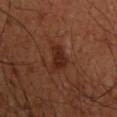| field | value |
|---|---|
| biopsy status | total-body-photography surveillance lesion; no biopsy |
| anatomic site | the left upper arm |
| image | total-body-photography crop, ~15 mm field of view |
| lesion diameter | ≈3.5 mm |
| illumination | cross-polarized |
| subject | male, aged approximately 50 |